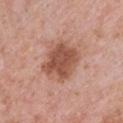Impression:
No biopsy was performed on this lesion — it was imaged during a full skin examination and was not determined to be concerning.